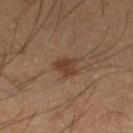* notes — total-body-photography surveillance lesion; no biopsy
* subject — male, aged approximately 40
* image — ~15 mm crop, total-body skin-cancer survey
* body site — the left lower leg
* image-analysis metrics — a lesion color around L≈38 a*≈19 b*≈27 in CIELAB and about 9 CIELAB-L* units darker than the surrounding skin; border irregularity of about 4.5 on a 0–10 scale, a within-lesion color-variation index near 2/10, and a peripheral color-asymmetry measure near 0.5; a classifier nevus-likeness of about 85/100 and a detector confidence of about 100 out of 100 that the crop contains a lesion
* lighting — cross-polarized illumination
* lesion size — ~3 mm (longest diameter)